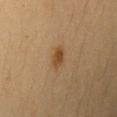Case summary:
- workup — total-body-photography surveillance lesion; no biopsy
- subject — female, aged approximately 30
- image source — total-body-photography crop, ~15 mm field of view
- tile lighting — cross-polarized illumination
- body site — the right upper arm